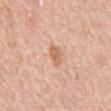• workup — total-body-photography surveillance lesion; no biopsy
• image — ~15 mm tile from a whole-body skin photo
• lesion diameter — ≈2.5 mm
• lighting — white-light
• body site — the mid back
• subject — female, roughly 65 years of age